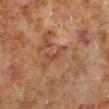Q: Is there a histopathology result?
A: imaged on a skin check; not biopsied
Q: How large is the lesion?
A: about 3.5 mm
Q: Where on the body is the lesion?
A: the left lower leg
Q: What is the imaging modality?
A: total-body-photography crop, ~15 mm field of view
Q: Automated lesion metrics?
A: a footprint of about 3.5 mm², a shape eccentricity near 0.95, and a shape-asymmetry score of about 0.65 (0 = symmetric)
Q: How was the tile lit?
A: cross-polarized illumination
Q: What are the patient's age and sex?
A: male, aged 58 to 62